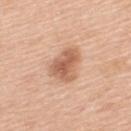{
  "biopsy_status": "not biopsied; imaged during a skin examination",
  "lighting": "white-light",
  "image": {
    "source": "total-body photography crop",
    "field_of_view_mm": 15
  },
  "lesion_size": {
    "long_diameter_mm_approx": 4.0
  },
  "automated_metrics": {
    "eccentricity": 0.65,
    "shape_asymmetry": 0.4,
    "cielab_L": 60,
    "cielab_a": 22,
    "cielab_b": 33,
    "vs_skin_darker_L": 12.0,
    "vs_skin_contrast_norm": 8.0,
    "border_irregularity_0_10": 3.5,
    "color_variation_0_10": 4.5
  },
  "patient": {
    "sex": "male",
    "age_approx": 50
  },
  "site": "upper back"
}